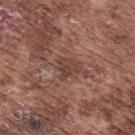The lesion was photographed on a routine skin check and not biopsied; there is no pathology result.
Approximately 3 mm at its widest.
Cropped from a total-body skin-imaging series; the visible field is about 15 mm.
The patient is a male aged 73 to 77.
The lesion is located on the upper back.